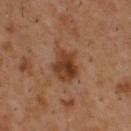| feature | finding |
|---|---|
| workup | total-body-photography surveillance lesion; no biopsy |
| size | ~3.5 mm (longest diameter) |
| automated metrics | a shape eccentricity near 0.5 and two-axis asymmetry of about 0.25; a lesion color around L≈38 a*≈21 b*≈31 in CIELAB, a lesion–skin lightness drop of about 10, and a normalized border contrast of about 9; border irregularity of about 3 on a 0–10 scale and peripheral color asymmetry of about 2; a classifier nevus-likeness of about 90/100 and a detector confidence of about 100 out of 100 that the crop contains a lesion |
| patient | male, aged 53 to 57 |
| imaging modality | 15 mm crop, total-body photography |
| body site | the upper back |